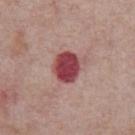Findings:
– notes — no biopsy performed (imaged during a skin exam)
– image — ~15 mm crop, total-body skin-cancer survey
– location — the chest
– automated lesion analysis — a footprint of about 10 mm² and a symmetry-axis asymmetry near 0.15; a border-irregularity index near 1.5/10, a within-lesion color-variation index near 4/10, and peripheral color asymmetry of about 1
– size — about 3.5 mm
– tile lighting — white-light
– subject — male, aged around 75